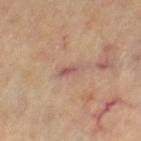| feature | finding |
|---|---|
| follow-up | catalogued during a skin exam; not biopsied |
| subject | female, roughly 60 years of age |
| tile lighting | cross-polarized |
| site | the leg |
| imaging modality | ~15 mm crop, total-body skin-cancer survey |